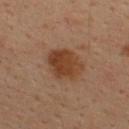Assessment:
Part of a total-body skin-imaging series; this lesion was reviewed on a skin check and was not flagged for biopsy.
Acquisition and patient details:
This is a cross-polarized tile. The lesion's longest dimension is about 4 mm. The lesion-visualizer software estimated a lesion color around L≈36 a*≈20 b*≈29 in CIELAB and a normalized lesion–skin contrast near 9. It also reported a classifier nevus-likeness of about 90/100. Cropped from a whole-body photographic skin survey; the tile spans about 15 mm. A male subject approximately 35 years of age. On the upper back.